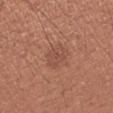No biopsy was performed on this lesion — it was imaged during a full skin examination and was not determined to be concerning. From the left forearm. The recorded lesion diameter is about 3.5 mm. Imaged with white-light lighting. A 15 mm close-up tile from a total-body photography series done for melanoma screening. A female subject, about 25 years old.